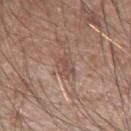| field | value |
|---|---|
| workup | imaged on a skin check; not biopsied |
| lesion diameter | ~3.5 mm (longest diameter) |
| subject | male, in their 60s |
| site | the right upper arm |
| tile lighting | white-light illumination |
| image-analysis metrics | a footprint of about 5.5 mm², a shape eccentricity near 0.75, and a shape-asymmetry score of about 0.25 (0 = symmetric); about 8 CIELAB-L* units darker than the surrounding skin and a normalized lesion–skin contrast near 6; border irregularity of about 2.5 on a 0–10 scale and peripheral color asymmetry of about 1; a nevus-likeness score of about 0/100 and a detector confidence of about 75 out of 100 that the crop contains a lesion |
| image | ~15 mm tile from a whole-body skin photo |